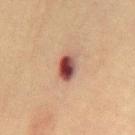Recorded during total-body skin imaging; not selected for excision or biopsy.